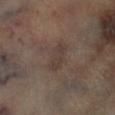Impression: Captured during whole-body skin photography for melanoma surveillance; the lesion was not biopsied. Context: On the left lower leg. A male patient about 65 years old. Longest diameter approximately 4 mm. An algorithmic analysis of the crop reported a border-irregularity index near 3/10, a color-variation rating of about 2/10, and peripheral color asymmetry of about 0.5. The software also gave a classifier nevus-likeness of about 0/100. A lesion tile, about 15 mm wide, cut from a 3D total-body photograph.